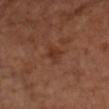Q: Is there a histopathology result?
A: total-body-photography surveillance lesion; no biopsy
Q: Lesion location?
A: the arm
Q: Illumination type?
A: cross-polarized
Q: How was this image acquired?
A: total-body-photography crop, ~15 mm field of view
Q: What are the patient's age and sex?
A: male, aged approximately 55